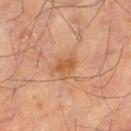notes — catalogued during a skin exam; not biopsied | image source — total-body-photography crop, ~15 mm field of view | anatomic site — the left thigh | patient — male, about 55 years old | lesion diameter — about 2.5 mm.